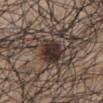- biopsy status — imaged on a skin check; not biopsied
- size — about 4.5 mm
- anatomic site — the mid back
- tile lighting — white-light illumination
- image source — total-body-photography crop, ~15 mm field of view
- patient — male, aged approximately 70